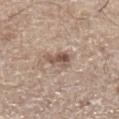This is a white-light tile.
Located on the left lower leg.
The subject is a male in their mid-60s.
A 15 mm crop from a total-body photograph taken for skin-cancer surveillance.
The recorded lesion diameter is about 3 mm.
Automated tile analysis of the lesion measured a lesion area of about 5 mm², an eccentricity of roughly 0.75, and a symmetry-axis asymmetry near 0.4. It also reported a mean CIELAB color near L≈53 a*≈16 b*≈25 and a lesion-to-skin contrast of about 8 (normalized; higher = more distinct). The analysis additionally found a detector confidence of about 100 out of 100 that the crop contains a lesion.